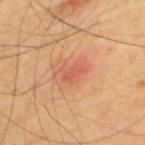Captured during whole-body skin photography for melanoma surveillance; the lesion was not biopsied.
On the upper back.
The tile uses cross-polarized illumination.
Longest diameter approximately 3 mm.
A roughly 15 mm field-of-view crop from a total-body skin photograph.
The subject is a male aged around 65.